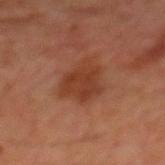Imaged during a routine full-body skin examination; the lesion was not biopsied and no histopathology is available.
Cropped from a total-body skin-imaging series; the visible field is about 15 mm.
From the chest.
The patient is a male approximately 60 years of age.
Approximately 4 mm at its widest.
The total-body-photography lesion software estimated a nevus-likeness score of about 40/100 and a lesion-detection confidence of about 100/100.
This is a cross-polarized tile.